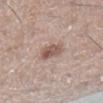{"biopsy_status": "not biopsied; imaged during a skin examination", "image": {"source": "total-body photography crop", "field_of_view_mm": 15}, "lesion_size": {"long_diameter_mm_approx": 3.5}, "patient": {"sex": "female", "age_approx": 60}, "site": "right lower leg", "lighting": "white-light"}